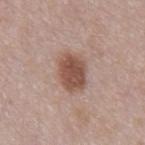Part of a total-body skin-imaging series; this lesion was reviewed on a skin check and was not flagged for biopsy. The total-body-photography lesion software estimated a footprint of about 10 mm², a shape eccentricity near 0.7, and two-axis asymmetry of about 0.15. It also reported a lesion color around L≈50 a*≈21 b*≈26 in CIELAB, roughly 13 lightness units darker than nearby skin, and a normalized lesion–skin contrast near 9.5. The software also gave a nevus-likeness score of about 100/100. Longest diameter approximately 4 mm. Captured under white-light illumination. A roughly 15 mm field-of-view crop from a total-body skin photograph. The lesion is on the abdomen. A male patient in their mid- to late 50s.